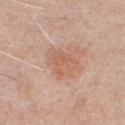Q: Was a biopsy performed?
A: total-body-photography surveillance lesion; no biopsy
Q: What did automated image analysis measure?
A: a footprint of about 7 mm², an outline eccentricity of about 0.6 (0 = round, 1 = elongated), and a symmetry-axis asymmetry near 0.45; border irregularity of about 5.5 on a 0–10 scale and radial color variation of about 0.5
Q: How large is the lesion?
A: about 3.5 mm
Q: What lighting was used for the tile?
A: white-light
Q: What is the imaging modality?
A: 15 mm crop, total-body photography
Q: What are the patient's age and sex?
A: male, aged 58–62
Q: What is the anatomic site?
A: the chest